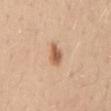notes: total-body-photography surveillance lesion; no biopsy
lesion size: ~3 mm (longest diameter)
acquisition: total-body-photography crop, ~15 mm field of view
site: the mid back
patient: female, roughly 45 years of age
lighting: white-light illumination